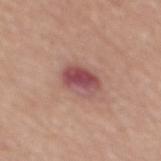Recorded during total-body skin imaging; not selected for excision or biopsy. A close-up tile cropped from a whole-body skin photograph, about 15 mm across. Longest diameter approximately 4 mm. A female patient, aged 53–57. Captured under white-light illumination. An algorithmic analysis of the crop reported an eccentricity of roughly 0.7 and a shape-asymmetry score of about 0.15 (0 = symmetric). And it measured an automated nevus-likeness rating near 15 out of 100. From the mid back.